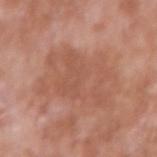Clinical impression:
Part of a total-body skin-imaging series; this lesion was reviewed on a skin check and was not flagged for biopsy.
Background:
Automated tile analysis of the lesion measured a lesion color around L≈55 a*≈23 b*≈30 in CIELAB, roughly 6 lightness units darker than nearby skin, and a normalized border contrast of about 4.5. The software also gave a peripheral color-asymmetry measure near 1. The software also gave a nevus-likeness score of about 0/100 and a lesion-detection confidence of about 100/100. A male subject aged around 60. Measured at roughly 8 mm in maximum diameter. This is a white-light tile. The lesion is on the right upper arm. A lesion tile, about 15 mm wide, cut from a 3D total-body photograph.